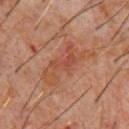Impression:
Imaged during a routine full-body skin examination; the lesion was not biopsied and no histopathology is available.
Clinical summary:
A 15 mm close-up tile from a total-body photography series done for melanoma screening. The patient is a male about 60 years old. The recorded lesion diameter is about 7 mm. Imaged with cross-polarized lighting. From the chest.